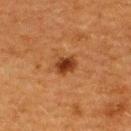Notes:
– notes: imaged on a skin check; not biopsied
– subject: female, about 40 years old
– lighting: cross-polarized illumination
– site: the upper back
– lesion diameter: about 2.5 mm
– acquisition: ~15 mm crop, total-body skin-cancer survey